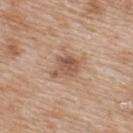Clinical impression: The lesion was photographed on a routine skin check and not biopsied; there is no pathology result. Clinical summary: Captured under white-light illumination. Measured at roughly 3.5 mm in maximum diameter. The lesion is on the back. A roughly 15 mm field-of-view crop from a total-body skin photograph. The subject is a female about 40 years old.